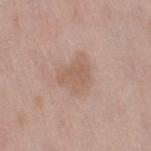Imaged during a routine full-body skin examination; the lesion was not biopsied and no histopathology is available.
This image is a 15 mm lesion crop taken from a total-body photograph.
About 4 mm across.
A female subject in their 50s.
The lesion is on the right thigh.